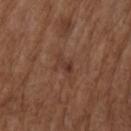Clinical impression: Part of a total-body skin-imaging series; this lesion was reviewed on a skin check and was not flagged for biopsy. Acquisition and patient details: The lesion's longest dimension is about 3 mm. A male subject, aged 73–77. On the right upper arm. A region of skin cropped from a whole-body photographic capture, roughly 15 mm wide.